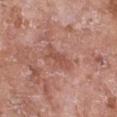Q: Was this lesion biopsied?
A: imaged on a skin check; not biopsied
Q: Where on the body is the lesion?
A: the chest
Q: What lighting was used for the tile?
A: white-light
Q: Who is the patient?
A: female, approximately 75 years of age
Q: How was this image acquired?
A: ~15 mm crop, total-body skin-cancer survey